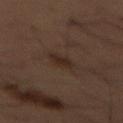Notes:
- site · the abdomen
- lighting · cross-polarized
- imaging modality · 15 mm crop, total-body photography
- diameter · about 3 mm
- patient · male, aged around 55